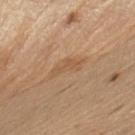Notes:
- workup — no biopsy performed (imaged during a skin exam)
- tile lighting — white-light
- automated lesion analysis — an area of roughly 4.5 mm² and two-axis asymmetry of about 0.5; a mean CIELAB color near L≈55 a*≈19 b*≈34 and a lesion-to-skin contrast of about 5 (normalized; higher = more distinct)
- anatomic site — the left upper arm
- patient — male, about 70 years old
- lesion diameter — ~4 mm (longest diameter)
- imaging modality — ~15 mm crop, total-body skin-cancer survey